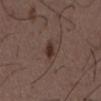Q: Is there a histopathology result?
A: no biopsy performed (imaged during a skin exam)
Q: Where on the body is the lesion?
A: the mid back
Q: Patient demographics?
A: male, aged 48 to 52
Q: How was this image acquired?
A: ~15 mm crop, total-body skin-cancer survey
Q: Lesion size?
A: about 2.5 mm
Q: Automated lesion metrics?
A: an area of roughly 3 mm², an outline eccentricity of about 0.85 (0 = round, 1 = elongated), and two-axis asymmetry of about 0.2; about 10 CIELAB-L* units darker than the surrounding skin and a normalized border contrast of about 10; a within-lesion color-variation index near 1.5/10 and a peripheral color-asymmetry measure near 0.5; a classifier nevus-likeness of about 95/100
Q: What lighting was used for the tile?
A: white-light